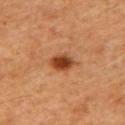Findings:
* tile lighting · cross-polarized
* image · ~15 mm crop, total-body skin-cancer survey
* body site · the mid back
* subject · male, about 60 years old